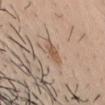This lesion was catalogued during total-body skin photography and was not selected for biopsy.
A 15 mm crop from a total-body photograph taken for skin-cancer surveillance.
On the head or neck.
The subject is a male aged around 25.
The lesion's longest dimension is about 3 mm.